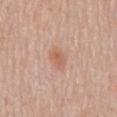Captured during whole-body skin photography for melanoma surveillance; the lesion was not biopsied. A lesion tile, about 15 mm wide, cut from a 3D total-body photograph. Measured at roughly 3 mm in maximum diameter. The lesion-visualizer software estimated an outline eccentricity of about 0.8 (0 = round, 1 = elongated) and a shape-asymmetry score of about 0.2 (0 = symmetric). The software also gave a mean CIELAB color near L≈61 a*≈21 b*≈31, about 8 CIELAB-L* units darker than the surrounding skin, and a normalized border contrast of about 6. Imaged with white-light lighting. On the mid back. A male subject, approximately 80 years of age.